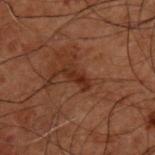* follow-up · total-body-photography surveillance lesion; no biopsy
* image source · 15 mm crop, total-body photography
* size · ≈3 mm
* patient · male, in their 50s
* TBP lesion metrics · an eccentricity of roughly 0.95 and a shape-asymmetry score of about 0.3 (0 = symmetric); an average lesion color of about L≈21 a*≈19 b*≈24 (CIELAB), roughly 7 lightness units darker than nearby skin, and a lesion-to-skin contrast of about 8 (normalized; higher = more distinct); a classifier nevus-likeness of about 55/100 and a lesion-detection confidence of about 100/100
* anatomic site · the back
* illumination · cross-polarized